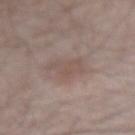Clinical impression:
Recorded during total-body skin imaging; not selected for excision or biopsy.
Acquisition and patient details:
The subject is a male approximately 55 years of age. Automated tile analysis of the lesion measured an eccentricity of roughly 0.65. The analysis additionally found roughly 6 lightness units darker than nearby skin and a lesion-to-skin contrast of about 5 (normalized; higher = more distinct). The software also gave a border-irregularity index near 4.5/10, a within-lesion color-variation index near 3.5/10, and peripheral color asymmetry of about 1. And it measured a nevus-likeness score of about 30/100 and a detector confidence of about 100 out of 100 that the crop contains a lesion. The lesion is located on the arm. A 15 mm crop from a total-body photograph taken for skin-cancer surveillance. Captured under white-light illumination. Longest diameter approximately 3.5 mm.